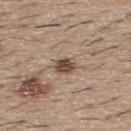Clinical impression: Part of a total-body skin-imaging series; this lesion was reviewed on a skin check and was not flagged for biopsy. Clinical summary: Located on the upper back. A 15 mm close-up tile from a total-body photography series done for melanoma screening. A male subject roughly 60 years of age.